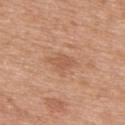Imaged during a routine full-body skin examination; the lesion was not biopsied and no histopathology is available. A region of skin cropped from a whole-body photographic capture, roughly 15 mm wide. The lesion is on the upper back. A male patient, roughly 50 years of age.